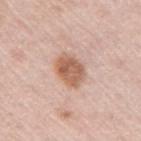Notes:
• patient · female, aged 63 to 67
• location · the right upper arm
• image · 15 mm crop, total-body photography
• size · ~4 mm (longest diameter)
• tile lighting · white-light